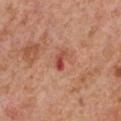Recorded during total-body skin imaging; not selected for excision or biopsy. Imaged with white-light lighting. A roughly 15 mm field-of-view crop from a total-body skin photograph. The lesion is located on the front of the torso. The subject is a male roughly 65 years of age.